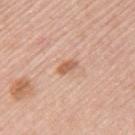Part of a total-body skin-imaging series; this lesion was reviewed on a skin check and was not flagged for biopsy.
From the left upper arm.
The lesion's longest dimension is about 2.5 mm.
A female subject aged 63 to 67.
A roughly 15 mm field-of-view crop from a total-body skin photograph.